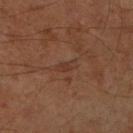The lesion was tiled from a total-body skin photograph and was not biopsied. Longest diameter approximately 2.5 mm. A 15 mm close-up extracted from a 3D total-body photography capture. A male subject about 60 years old. The lesion is located on the right lower leg. The total-body-photography lesion software estimated peripheral color asymmetry of about 0. And it measured a classifier nevus-likeness of about 0/100 and a lesion-detection confidence of about 90/100.